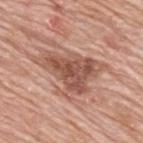biopsy status: imaged on a skin check; not biopsied | location: the upper back | lesion size: ≈8 mm | illumination: white-light illumination | subject: male, in their 80s | acquisition: 15 mm crop, total-body photography.